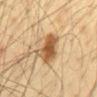Clinical impression:
No biopsy was performed on this lesion — it was imaged during a full skin examination and was not determined to be concerning.
Clinical summary:
A roughly 15 mm field-of-view crop from a total-body skin photograph. This is a cross-polarized tile. The total-body-photography lesion software estimated a shape eccentricity near 0.75. The software also gave a lesion color around L≈52 a*≈18 b*≈35 in CIELAB, a lesion–skin lightness drop of about 13, and a lesion-to-skin contrast of about 9.5 (normalized; higher = more distinct). The analysis additionally found border irregularity of about 3.5 on a 0–10 scale. It also reported an automated nevus-likeness rating near 95 out of 100 and a lesion-detection confidence of about 100/100. Measured at roughly 5 mm in maximum diameter. The subject is a male aged 33–37. The lesion is located on the abdomen.